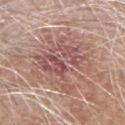follow-up — catalogued during a skin exam; not biopsied | site — the right forearm | automated metrics — a footprint of about 32 mm², a shape eccentricity near 0.45, and a shape-asymmetry score of about 0.35 (0 = symmetric); a lesion color around L≈55 a*≈21 b*≈22 in CIELAB and a normalized lesion–skin contrast near 7; border irregularity of about 7.5 on a 0–10 scale and internal color variation of about 7.5 on a 0–10 scale | acquisition — ~15 mm crop, total-body skin-cancer survey | patient — male, aged 63–67 | lesion size — ≈7.5 mm | lighting — white-light illumination.